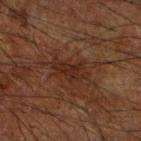No biopsy was performed on this lesion — it was imaged during a full skin examination and was not determined to be concerning.
Located on the right forearm.
A region of skin cropped from a whole-body photographic capture, roughly 15 mm wide.
A male patient roughly 65 years of age.